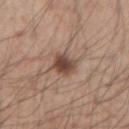Clinical impression:
The lesion was tiled from a total-body skin photograph and was not biopsied.
Background:
A male subject aged around 35. The lesion is located on the left forearm. A lesion tile, about 15 mm wide, cut from a 3D total-body photograph.